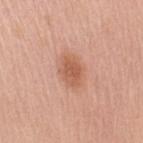Part of a total-body skin-imaging series; this lesion was reviewed on a skin check and was not flagged for biopsy.
A female subject, about 60 years old.
A 15 mm close-up tile from a total-body photography series done for melanoma screening.
The recorded lesion diameter is about 3.5 mm.
Automated tile analysis of the lesion measured an area of roughly 7.5 mm² and a shape eccentricity near 0.7. The software also gave a lesion–skin lightness drop of about 10 and a lesion-to-skin contrast of about 7 (normalized; higher = more distinct). The analysis additionally found a border-irregularity rating of about 2/10 and peripheral color asymmetry of about 1.
From the right upper arm.
Imaged with white-light lighting.